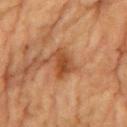{"image": {"source": "total-body photography crop", "field_of_view_mm": 15}, "site": "chest", "lesion_size": {"long_diameter_mm_approx": 3.5}, "patient": {"sex": "male", "age_approx": "85+"}}